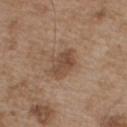Clinical impression:
Recorded during total-body skin imaging; not selected for excision or biopsy.
Acquisition and patient details:
A close-up tile cropped from a whole-body skin photograph, about 15 mm across. A male subject in their mid- to late 50s. The lesion is located on the front of the torso. The lesion-visualizer software estimated a color-variation rating of about 3.5/10 and radial color variation of about 1. The tile uses white-light illumination. Measured at roughly 4 mm in maximum diameter.